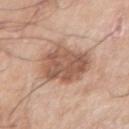<record>
  <biopsy_status>not biopsied; imaged during a skin examination</biopsy_status>
  <site>right upper arm</site>
  <image>
    <source>total-body photography crop</source>
    <field_of_view_mm>15</field_of_view_mm>
  </image>
  <lighting>white-light</lighting>
  <automated_metrics>
    <area_mm2_approx>22.0</area_mm2_approx>
    <eccentricity>0.8</eccentricity>
    <shape_asymmetry>0.2</shape_asymmetry>
  </automated_metrics>
  <patient>
    <sex>male</sex>
    <age_approx>65</age_approx>
  </patient>
  <lesion_size>
    <long_diameter_mm_approx>7.0</long_diameter_mm_approx>
  </lesion_size>
</record>